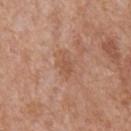size: ~3 mm (longest diameter)
patient: male, roughly 65 years of age
lighting: white-light illumination
TBP lesion metrics: a lesion color around L≈54 a*≈22 b*≈31 in CIELAB, a lesion–skin lightness drop of about 7, and a lesion-to-skin contrast of about 5.5 (normalized; higher = more distinct)
anatomic site: the abdomen
image: total-body-photography crop, ~15 mm field of view